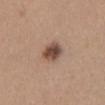Clinical impression:
Part of a total-body skin-imaging series; this lesion was reviewed on a skin check and was not flagged for biopsy.
Image and clinical context:
A 15 mm close-up tile from a total-body photography series done for melanoma screening. The lesion is located on the lower back. The patient is a female aged 43–47.